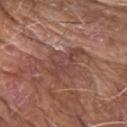This lesion was catalogued during total-body skin photography and was not selected for biopsy. A close-up tile cropped from a whole-body skin photograph, about 15 mm across. The lesion is located on the right forearm. The subject is a male aged 73–77.